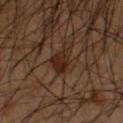Case summary:
- follow-up: imaged on a skin check; not biopsied
- diameter: ≈3 mm
- patient: male, aged 48 to 52
- image source: total-body-photography crop, ~15 mm field of view
- automated metrics: a border-irregularity rating of about 3/10 and radial color variation of about 1.5; a classifier nevus-likeness of about 55/100
- body site: the left forearm